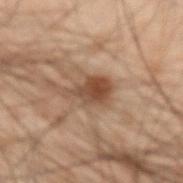The lesion was photographed on a routine skin check and not biopsied; there is no pathology result. Captured under cross-polarized illumination. Automated image analysis of the tile measured a mean CIELAB color near L≈35 a*≈14 b*≈23 and a lesion–skin lightness drop of about 9. The lesion is on the left forearm. Longest diameter approximately 4.5 mm. The patient is a male aged around 50. Cropped from a total-body skin-imaging series; the visible field is about 15 mm.